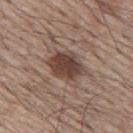<lesion>
<biopsy_status>not biopsied; imaged during a skin examination</biopsy_status>
<lesion_size>
  <long_diameter_mm_approx>4.5</long_diameter_mm_approx>
</lesion_size>
<site>mid back</site>
<patient>
  <sex>male</sex>
  <age_approx>65</age_approx>
</patient>
<image>
  <source>total-body photography crop</source>
  <field_of_view_mm>15</field_of_view_mm>
</image>
</lesion>